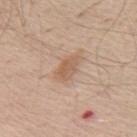Part of a total-body skin-imaging series; this lesion was reviewed on a skin check and was not flagged for biopsy.
The lesion's longest dimension is about 3.5 mm.
The patient is a male approximately 60 years of age.
This image is a 15 mm lesion crop taken from a total-body photograph.
From the chest.
The tile uses white-light illumination.